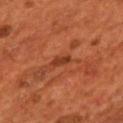No biopsy was performed on this lesion — it was imaged during a full skin examination and was not determined to be concerning.
A male subject, about 55 years old.
Cropped from a total-body skin-imaging series; the visible field is about 15 mm.
Located on the chest.